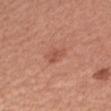Part of a total-body skin-imaging series; this lesion was reviewed on a skin check and was not flagged for biopsy.